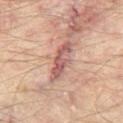workup = catalogued during a skin exam; not biopsied | size = about 5 mm | site = the leg | illumination = cross-polarized | subject = roughly 55 years of age | image source = ~15 mm crop, total-body skin-cancer survey | automated metrics = a lesion area of about 7 mm², an eccentricity of roughly 0.95, and two-axis asymmetry of about 0.3; border irregularity of about 5 on a 0–10 scale, a within-lesion color-variation index near 2/10, and a peripheral color-asymmetry measure near 0.5; a classifier nevus-likeness of about 0/100 and a detector confidence of about 70 out of 100 that the crop contains a lesion.